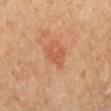biopsy status — catalogued during a skin exam; not biopsied | anatomic site — the left forearm | image-analysis metrics — a lesion area of about 4 mm² and an outline eccentricity of about 0.75 (0 = round, 1 = elongated); a mean CIELAB color near L≈56 a*≈28 b*≈35, about 7 CIELAB-L* units darker than the surrounding skin, and a normalized border contrast of about 5; a nevus-likeness score of about 50/100 and a lesion-detection confidence of about 100/100 | diameter — ≈3 mm | patient — female, roughly 65 years of age | illumination — cross-polarized illumination | image source — 15 mm crop, total-body photography.